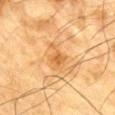| key | value |
|---|---|
| lesion size | ≈3.5 mm |
| subject | male, in their mid- to late 80s or older |
| image-analysis metrics | a lesion–skin lightness drop of about 8 and a lesion-to-skin contrast of about 5.5 (normalized; higher = more distinct); a color-variation rating of about 3/10 and radial color variation of about 1; an automated nevus-likeness rating near 0 out of 100 |
| lighting | cross-polarized |
| anatomic site | the front of the torso |
| acquisition | ~15 mm crop, total-body skin-cancer survey |